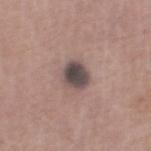follow-up = imaged on a skin check; not biopsied
subject = male, in their mid-60s
image source = total-body-photography crop, ~15 mm field of view
location = the right lower leg